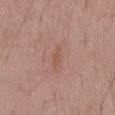Impression: No biopsy was performed on this lesion — it was imaged during a full skin examination and was not determined to be concerning. Clinical summary: A male patient aged 53–57. An algorithmic analysis of the crop reported an area of roughly 2 mm², an eccentricity of roughly 0.9, and a shape-asymmetry score of about 0.4 (0 = symmetric). And it measured a color-variation rating of about 0/10 and peripheral color asymmetry of about 0. It also reported an automated nevus-likeness rating near 0 out of 100 and a lesion-detection confidence of about 100/100. Imaged with white-light lighting. The lesion is on the mid back. A roughly 15 mm field-of-view crop from a total-body skin photograph.